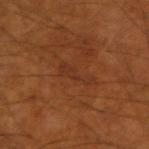Clinical impression:
The lesion was tiled from a total-body skin photograph and was not biopsied.